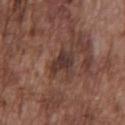follow-up: catalogued during a skin exam; not biopsied | patient: male, aged 73–77 | image source: total-body-photography crop, ~15 mm field of view | diameter: about 3 mm | site: the chest.